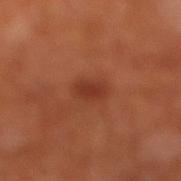Part of a total-body skin-imaging series; this lesion was reviewed on a skin check and was not flagged for biopsy. A male patient, aged 68 to 72. This is a cross-polarized tile. Measured at roughly 2.5 mm in maximum diameter. The lesion is on the leg. This image is a 15 mm lesion crop taken from a total-body photograph. The total-body-photography lesion software estimated border irregularity of about 1.5 on a 0–10 scale and radial color variation of about 0.5. It also reported lesion-presence confidence of about 100/100.